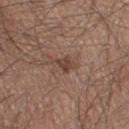Assessment: The lesion was tiled from a total-body skin photograph and was not biopsied. Image and clinical context: Measured at roughly 3 mm in maximum diameter. A region of skin cropped from a whole-body photographic capture, roughly 15 mm wide. A male subject approximately 45 years of age. Imaged with white-light lighting. The lesion is located on the leg. Automated image analysis of the tile measured an average lesion color of about L≈43 a*≈18 b*≈25 (CIELAB) and a lesion–skin lightness drop of about 8.